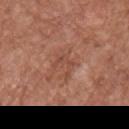The subject is a male aged around 75.
Located on the chest.
A close-up tile cropped from a whole-body skin photograph, about 15 mm across.